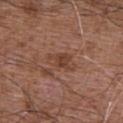Clinical summary:
Captured under white-light illumination. Located on the abdomen. The lesion-visualizer software estimated a mean CIELAB color near L≈42 a*≈22 b*≈28 and a lesion-to-skin contrast of about 7 (normalized; higher = more distinct). And it measured border irregularity of about 4.5 on a 0–10 scale and radial color variation of about 0.5. A male subject, aged 73–77. Cropped from a total-body skin-imaging series; the visible field is about 15 mm.